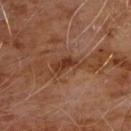Clinical impression: Imaged during a routine full-body skin examination; the lesion was not biopsied and no histopathology is available. Background: An algorithmic analysis of the crop reported a footprint of about 6.5 mm², a shape eccentricity near 0.9, and a shape-asymmetry score of about 0.55 (0 = symmetric). And it measured a lesion color around L≈36 a*≈21 b*≈29 in CIELAB, roughly 8 lightness units darker than nearby skin, and a lesion-to-skin contrast of about 7.5 (normalized; higher = more distinct). The analysis additionally found a border-irregularity index near 7.5/10, a within-lesion color-variation index near 3/10, and a peripheral color-asymmetry measure near 1. Measured at roughly 5 mm in maximum diameter. A 15 mm close-up tile from a total-body photography series done for melanoma screening. A male patient aged 58–62. On the chest. Captured under cross-polarized illumination.